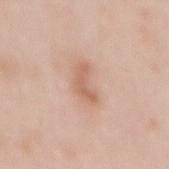Impression:
This lesion was catalogued during total-body skin photography and was not selected for biopsy.
Clinical summary:
A female patient aged 38 to 42. A 15 mm close-up tile from a total-body photography series done for melanoma screening. On the back.